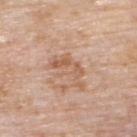Q: Where on the body is the lesion?
A: the upper back
Q: What are the patient's age and sex?
A: male, in their mid-70s
Q: What kind of image is this?
A: total-body-photography crop, ~15 mm field of view